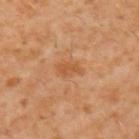Q: Is there a histopathology result?
A: catalogued during a skin exam; not biopsied
Q: Illumination type?
A: cross-polarized
Q: What is the imaging modality?
A: total-body-photography crop, ~15 mm field of view
Q: What are the patient's age and sex?
A: male, aged approximately 60
Q: Lesion size?
A: ~3 mm (longest diameter)
Q: Where on the body is the lesion?
A: the upper back